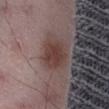notes = total-body-photography surveillance lesion; no biopsy
imaging modality = 15 mm crop, total-body photography
subject = male, in their 50s
location = the right thigh